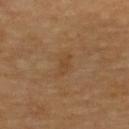biopsy status = no biopsy performed (imaged during a skin exam) | acquisition = ~15 mm crop, total-body skin-cancer survey | patient = aged 58 to 62 | site = the upper back.